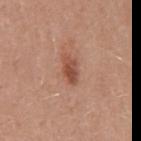Case summary:
* subject — male, aged 43 to 47
* image-analysis metrics — a lesion color around L≈50 a*≈24 b*≈31 in CIELAB, a lesion–skin lightness drop of about 11, and a normalized lesion–skin contrast near 8
* illumination — white-light
* site — the mid back
* image — ~15 mm crop, total-body skin-cancer survey
* size — ≈3.5 mm